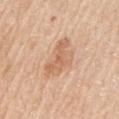Image and clinical context:
A male subject, aged 58 to 62. Approximately 5.5 mm at its widest. Captured under white-light illumination. A 15 mm crop from a total-body photograph taken for skin-cancer surveillance. Located on the arm. Automated tile analysis of the lesion measured an average lesion color of about L≈64 a*≈21 b*≈34 (CIELAB), roughly 9 lightness units darker than nearby skin, and a lesion-to-skin contrast of about 5.5 (normalized; higher = more distinct). The analysis additionally found a border-irregularity rating of about 5/10, a within-lesion color-variation index near 3/10, and a peripheral color-asymmetry measure near 1. And it measured an automated nevus-likeness rating near 10 out of 100.